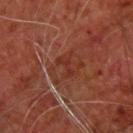follow-up — catalogued during a skin exam; not biopsied | body site — the back | subject — male, aged around 65 | image — ~15 mm crop, total-body skin-cancer survey.